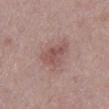follow-up: imaged on a skin check; not biopsied | automated metrics: a footprint of about 8.5 mm², an eccentricity of roughly 0.8, and two-axis asymmetry of about 0.4; a lesion color around L≈52 a*≈21 b*≈21 in CIELAB, about 9 CIELAB-L* units darker than the surrounding skin, and a normalized lesion–skin contrast near 6; a border-irregularity rating of about 4.5/10, internal color variation of about 3.5 on a 0–10 scale, and a peripheral color-asymmetry measure near 1.5 | subject: male, aged around 70 | imaging modality: ~15 mm tile from a whole-body skin photo | size: about 4.5 mm | location: the mid back | lighting: white-light illumination.